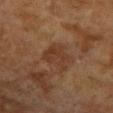The lesion was tiled from a total-body skin photograph and was not biopsied. A female patient, aged around 80. Imaged with cross-polarized lighting. Cropped from a whole-body photographic skin survey; the tile spans about 15 mm. The recorded lesion diameter is about 3.5 mm. Located on the arm.